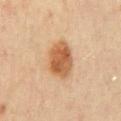{
  "biopsy_status": "not biopsied; imaged during a skin examination",
  "lesion_size": {
    "long_diameter_mm_approx": 4.5
  },
  "patient": {
    "sex": "male",
    "age_approx": 45
  },
  "automated_metrics": {
    "cielab_L": 49,
    "cielab_a": 20,
    "cielab_b": 34,
    "vs_skin_darker_L": 12.0,
    "vs_skin_contrast_norm": 9.5,
    "color_variation_0_10": 4.0,
    "peripheral_color_asymmetry": 1.0
  },
  "site": "mid back",
  "image": {
    "source": "total-body photography crop",
    "field_of_view_mm": 15
  }
}